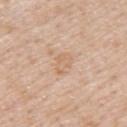This lesion was catalogued during total-body skin photography and was not selected for biopsy.
Measured at roughly 3 mm in maximum diameter.
The lesion is on the upper back.
The patient is a male about 50 years old.
A 15 mm crop from a total-body photograph taken for skin-cancer surveillance.
Imaged with white-light lighting.